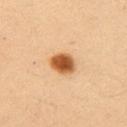Impression: This lesion was catalogued during total-body skin photography and was not selected for biopsy. Clinical summary: The lesion is located on the right upper arm. A roughly 15 mm field-of-view crop from a total-body skin photograph. Longest diameter approximately 3.5 mm. The subject is a female aged 33 to 37.